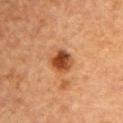Impression:
This lesion was catalogued during total-body skin photography and was not selected for biopsy.
Context:
From the chest. The recorded lesion diameter is about 3 mm. The tile uses cross-polarized illumination. A region of skin cropped from a whole-body photographic capture, roughly 15 mm wide. The patient is a female aged 68 to 72.